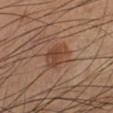• follow-up — imaged on a skin check; not biopsied
• image-analysis metrics — an average lesion color of about L≈42 a*≈20 b*≈29 (CIELAB) and about 9 CIELAB-L* units darker than the surrounding skin; border irregularity of about 2.5 on a 0–10 scale, a color-variation rating of about 3/10, and radial color variation of about 1
• body site — the leg
• image source — ~15 mm tile from a whole-body skin photo
• lesion size — ~4 mm (longest diameter)
• subject — male, aged 63 to 67